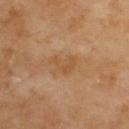Image and clinical context:
The lesion is on the upper back. A close-up tile cropped from a whole-body skin photograph, about 15 mm across.